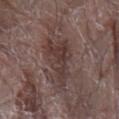The lesion was photographed on a routine skin check and not biopsied; there is no pathology result. About 6.5 mm across. Located on the right arm. A 15 mm crop from a total-body photograph taken for skin-cancer surveillance. A male patient aged around 80.